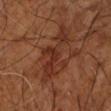Impression: No biopsy was performed on this lesion — it was imaged during a full skin examination and was not determined to be concerning. Image and clinical context: Imaged with cross-polarized lighting. The patient is aged 63–67. Located on the right upper arm. Approximately 7.5 mm at its widest. A 15 mm crop from a total-body photograph taken for skin-cancer surveillance.